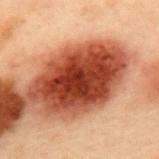{"biopsy_status": "not biopsied; imaged during a skin examination", "image": {"source": "total-body photography crop", "field_of_view_mm": 15}, "patient": {"sex": "male", "age_approx": 55}, "site": "upper back"}